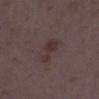| field | value |
|---|---|
| workup | catalogued during a skin exam; not biopsied |
| location | the leg |
| image source | 15 mm crop, total-body photography |
| subject | female, aged 33–37 |
| lighting | white-light |